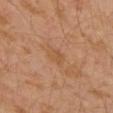Part of a total-body skin-imaging series; this lesion was reviewed on a skin check and was not flagged for biopsy. The lesion-visualizer software estimated roughly 5 lightness units darker than nearby skin and a normalized border contrast of about 5. It also reported a border-irregularity rating of about 2/10. Imaged with cross-polarized lighting. A lesion tile, about 15 mm wide, cut from a 3D total-body photograph. On the left arm. About 2.5 mm across. A male patient about 30 years old.